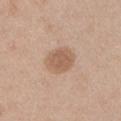biopsy_status: not biopsied; imaged during a skin examination
lesion_size:
  long_diameter_mm_approx: 3.5
automated_metrics:
  area_mm2_approx: 9.5
  eccentricity: 0.5
  shape_asymmetry: 0.15
  color_variation_0_10: 2.0
  nevus_likeness_0_100: 40
  lesion_detection_confidence_0_100: 100
lighting: white-light
site: arm
image:
  source: total-body photography crop
  field_of_view_mm: 15
patient:
  sex: male
  age_approx: 45